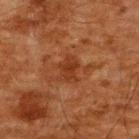Clinical impression: Recorded during total-body skin imaging; not selected for excision or biopsy. Image and clinical context: A male patient roughly 60 years of age. The tile uses cross-polarized illumination. A region of skin cropped from a whole-body photographic capture, roughly 15 mm wide. An algorithmic analysis of the crop reported an area of roughly 5.5 mm² and an eccentricity of roughly 0.75. The analysis additionally found a classifier nevus-likeness of about 0/100 and a lesion-detection confidence of about 100/100. The lesion is located on the upper back.